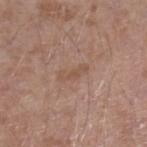Image and clinical context:
A male subject in their mid-40s. This is a white-light tile. About 3.5 mm across. Cropped from a whole-body photographic skin survey; the tile spans about 15 mm. The lesion is located on the right lower leg. Automated tile analysis of the lesion measured roughly 6 lightness units darker than nearby skin and a lesion-to-skin contrast of about 5 (normalized; higher = more distinct). And it measured border irregularity of about 4.5 on a 0–10 scale and a within-lesion color-variation index near 0/10.